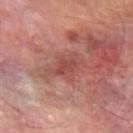No biopsy was performed on this lesion — it was imaged during a full skin examination and was not determined to be concerning. The subject is a male aged 68 to 72. The tile uses cross-polarized illumination. On the left forearm. The total-body-photography lesion software estimated a lesion color around L≈46 a*≈27 b*≈25 in CIELAB. The analysis additionally found border irregularity of about 4 on a 0–10 scale and radial color variation of about 1. Cropped from a whole-body photographic skin survey; the tile spans about 15 mm.